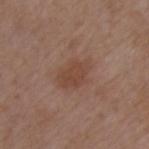workup: imaged on a skin check; not biopsied
tile lighting: white-light illumination
imaging modality: total-body-photography crop, ~15 mm field of view
subject: male, aged 48–52
location: the chest
TBP lesion metrics: a mean CIELAB color near L≈44 a*≈20 b*≈27; border irregularity of about 2.5 on a 0–10 scale, a within-lesion color-variation index near 2/10, and peripheral color asymmetry of about 0.5